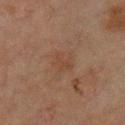follow-up: catalogued during a skin exam; not biopsied
tile lighting: cross-polarized illumination
TBP lesion metrics: a mean CIELAB color near L≈34 a*≈16 b*≈25 and a lesion–skin lightness drop of about 4; a border-irregularity index near 4/10, a within-lesion color-variation index near 1.5/10, and peripheral color asymmetry of about 0.5
anatomic site: the upper back
size: about 3 mm
subject: male, roughly 65 years of age
acquisition: 15 mm crop, total-body photography